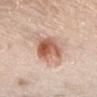notes = no biopsy performed (imaged during a skin exam).